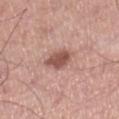Clinical impression:
Part of a total-body skin-imaging series; this lesion was reviewed on a skin check and was not flagged for biopsy.
Acquisition and patient details:
The lesion is located on the right lower leg. The lesion's longest dimension is about 3.5 mm. The lesion-visualizer software estimated an average lesion color of about L≈53 a*≈23 b*≈25 (CIELAB), roughly 13 lightness units darker than nearby skin, and a normalized border contrast of about 8.5. And it measured border irregularity of about 2.5 on a 0–10 scale, a color-variation rating of about 2.5/10, and radial color variation of about 1. And it measured a nevus-likeness score of about 90/100 and lesion-presence confidence of about 100/100. A 15 mm close-up tile from a total-body photography series done for melanoma screening. The subject is a male roughly 40 years of age. Imaged with white-light lighting.